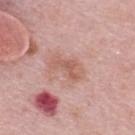| key | value |
|---|---|
| biopsy status | imaged on a skin check; not biopsied |
| anatomic site | the upper back |
| automated lesion analysis | about 8 CIELAB-L* units darker than the surrounding skin and a normalized lesion–skin contrast near 6 |
| imaging modality | total-body-photography crop, ~15 mm field of view |
| illumination | white-light |
| patient | female, in their mid-60s |